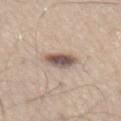Q: Was a biopsy performed?
A: imaged on a skin check; not biopsied
Q: How large is the lesion?
A: about 4 mm
Q: How was the tile lit?
A: white-light
Q: What are the patient's age and sex?
A: male, roughly 45 years of age
Q: What is the imaging modality?
A: ~15 mm tile from a whole-body skin photo
Q: What is the anatomic site?
A: the abdomen